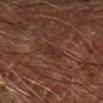Clinical impression: No biopsy was performed on this lesion — it was imaged during a full skin examination and was not determined to be concerning. Acquisition and patient details: The lesion is on the right forearm. A male subject, approximately 65 years of age. Cropped from a whole-body photographic skin survey; the tile spans about 15 mm.